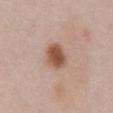Part of a total-body skin-imaging series; this lesion was reviewed on a skin check and was not flagged for biopsy.
The patient is a male aged around 55.
The lesion is located on the abdomen.
A lesion tile, about 15 mm wide, cut from a 3D total-body photograph.
Measured at roughly 3.5 mm in maximum diameter.